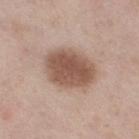Recorded during total-body skin imaging; not selected for excision or biopsy. Automated image analysis of the tile measured a footprint of about 21 mm² and an eccentricity of roughly 0.7. And it measured a border-irregularity index near 1.5/10 and internal color variation of about 3.5 on a 0–10 scale. From the leg. The lesion's longest dimension is about 6 mm. Captured under white-light illumination. A female patient, approximately 55 years of age. Cropped from a whole-body photographic skin survey; the tile spans about 15 mm.